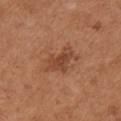Q: Lesion location?
A: the front of the torso
Q: Patient demographics?
A: female, approximately 65 years of age
Q: How was this image acquired?
A: total-body-photography crop, ~15 mm field of view
Q: What lighting was used for the tile?
A: white-light illumination
Q: What did automated image analysis measure?
A: a lesion area of about 7 mm², an eccentricity of roughly 0.8, and a symmetry-axis asymmetry near 0.35; an automated nevus-likeness rating near 20 out of 100 and lesion-presence confidence of about 100/100